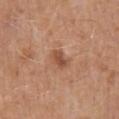Q: Is there a histopathology result?
A: no biopsy performed (imaged during a skin exam)
Q: What is the imaging modality?
A: ~15 mm tile from a whole-body skin photo
Q: Where on the body is the lesion?
A: the mid back
Q: What are the patient's age and sex?
A: male, about 70 years old
Q: What lighting was used for the tile?
A: white-light illumination
Q: Lesion size?
A: ≈2.5 mm